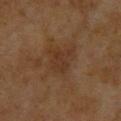biopsy_status: not biopsied; imaged during a skin examination
lesion_size:
  long_diameter_mm_approx: 3.5
site: back
lighting: cross-polarized
patient:
  sex: female
  age_approx: 60
image:
  source: total-body photography crop
  field_of_view_mm: 15
automated_metrics:
  area_mm2_approx: 7.0
  eccentricity: 0.65
  shape_asymmetry: 0.35
  cielab_L: 30
  cielab_a: 16
  cielab_b: 27
  vs_skin_darker_L: 5.0
  vs_skin_contrast_norm: 5.5